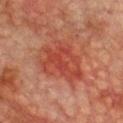biopsy status = catalogued during a skin exam; not biopsied
illumination = cross-polarized
anatomic site = the chest
lesion diameter = ~6 mm (longest diameter)
subject = male, approximately 75 years of age
acquisition = ~15 mm crop, total-body skin-cancer survey
automated metrics = an average lesion color of about L≈38 a*≈28 b*≈28 (CIELAB), roughly 7 lightness units darker than nearby skin, and a normalized border contrast of about 6; a border-irregularity rating of about 5/10, a color-variation rating of about 4.5/10, and peripheral color asymmetry of about 1.5